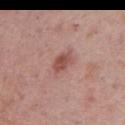Findings:
• follow-up: total-body-photography surveillance lesion; no biopsy
• subject: male, about 75 years old
• lesion size: about 3 mm
• imaging modality: ~15 mm tile from a whole-body skin photo
• tile lighting: white-light illumination
• location: the chest